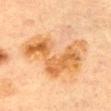Assessment: Captured during whole-body skin photography for melanoma surveillance; the lesion was not biopsied. Clinical summary: A roughly 15 mm field-of-view crop from a total-body skin photograph. The subject is a female aged 58 to 62. Located on the chest. The recorded lesion diameter is about 7.5 mm.